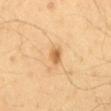Q: Was a biopsy performed?
A: catalogued during a skin exam; not biopsied
Q: What is the lesion's diameter?
A: ≈2 mm
Q: What lighting was used for the tile?
A: cross-polarized
Q: What is the imaging modality?
A: total-body-photography crop, ~15 mm field of view
Q: Patient demographics?
A: male, roughly 65 years of age
Q: Where on the body is the lesion?
A: the mid back